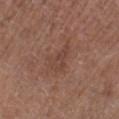Notes:
* biopsy status · no biopsy performed (imaged during a skin exam)
* tile lighting · white-light illumination
* automated lesion analysis · an average lesion color of about L≈43 a*≈19 b*≈25 (CIELAB), a lesion–skin lightness drop of about 6, and a lesion-to-skin contrast of about 5 (normalized; higher = more distinct); a nevus-likeness score of about 0/100 and a detector confidence of about 100 out of 100 that the crop contains a lesion
* patient · female, aged 78–82
* image source · total-body-photography crop, ~15 mm field of view
* lesion size · about 4.5 mm
* location · the right lower leg